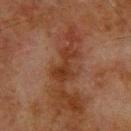  biopsy_status: not biopsied; imaged during a skin examination
  patient:
    sex: male
    age_approx: 65
  lighting: cross-polarized
  lesion_size:
    long_diameter_mm_approx: 4.5
  image:
    source: total-body photography crop
    field_of_view_mm: 15
  site: upper back
  automated_metrics:
    cielab_L: 28
    cielab_a: 20
    cielab_b: 27
    vs_skin_darker_L: 7.0
    vs_skin_contrast_norm: 8.0
    nevus_likeness_0_100: 0
    lesion_detection_confidence_0_100: 100